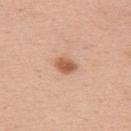<lesion>
  <patient>
    <sex>female</sex>
    <age_approx>35</age_approx>
  </patient>
  <lighting>white-light</lighting>
  <automated_metrics>
    <area_mm2_approx>4.0</area_mm2_approx>
    <eccentricity>0.7</eccentricity>
    <shape_asymmetry>0.3</shape_asymmetry>
    <cielab_L>58</cielab_L>
    <cielab_a>24</cielab_a>
    <cielab_b>34</cielab_b>
    <vs_skin_darker_L>13.0</vs_skin_darker_L>
    <border_irregularity_0_10>2.5</border_irregularity_0_10>
    <lesion_detection_confidence_0_100>100</lesion_detection_confidence_0_100>
  </automated_metrics>
  <image>
    <source>total-body photography crop</source>
    <field_of_view_mm>15</field_of_view_mm>
  </image>
  <site>upper back</site>
</lesion>